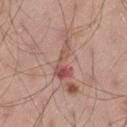Findings:
* notes · total-body-photography surveillance lesion; no biopsy
* lesion diameter · ≈4.5 mm
* body site · the left thigh
* image source · 15 mm crop, total-body photography
* patient · male, in their mid- to late 50s
* illumination · white-light illumination